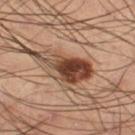notes: catalogued during a skin exam; not biopsied
body site: the right thigh
size: about 6.5 mm
subject: male, aged 53 to 57
image: 15 mm crop, total-body photography